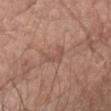• follow-up — total-body-photography surveillance lesion; no biopsy
• tile lighting — white-light
• subject — male, aged approximately 75
• lesion diameter — about 4 mm
• image source — ~15 mm crop, total-body skin-cancer survey
• site — the head or neck